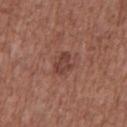No biopsy was performed on this lesion — it was imaged during a full skin examination and was not determined to be concerning. Automated tile analysis of the lesion measured an average lesion color of about L≈41 a*≈23 b*≈24 (CIELAB), a lesion–skin lightness drop of about 9, and a normalized border contrast of about 7. The software also gave an automated nevus-likeness rating near 10 out of 100 and a detector confidence of about 100 out of 100 that the crop contains a lesion. The lesion is located on the front of the torso. A male patient approximately 75 years of age. Cropped from a total-body skin-imaging series; the visible field is about 15 mm. Approximately 3 mm at its widest.